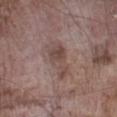Q: Was a biopsy performed?
A: catalogued during a skin exam; not biopsied
Q: How was this image acquired?
A: ~15 mm tile from a whole-body skin photo
Q: Lesion size?
A: about 4.5 mm
Q: Lesion location?
A: the left lower leg
Q: What are the patient's age and sex?
A: male, about 75 years old
Q: What lighting was used for the tile?
A: white-light illumination